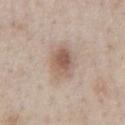{"biopsy_status": "not biopsied; imaged during a skin examination", "automated_metrics": {"cielab_L": 58, "cielab_a": 16, "cielab_b": 26, "vs_skin_darker_L": 11.0, "vs_skin_contrast_norm": 7.5, "nevus_likeness_0_100": 90}, "lighting": "white-light", "site": "chest", "image": {"source": "total-body photography crop", "field_of_view_mm": 15}, "lesion_size": {"long_diameter_mm_approx": 4.5}, "patient": {"sex": "male", "age_approx": 60}}